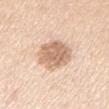This lesion was catalogued during total-body skin photography and was not selected for biopsy.
Cropped from a whole-body photographic skin survey; the tile spans about 15 mm.
Imaged with white-light lighting.
The lesion's longest dimension is about 4.5 mm.
A male subject, roughly 35 years of age.
The lesion is on the left upper arm.
Automated tile analysis of the lesion measured a lesion area of about 14 mm², an eccentricity of roughly 0.65, and a symmetry-axis asymmetry near 0.15. The software also gave a mean CIELAB color near L≈68 a*≈19 b*≈32, about 14 CIELAB-L* units darker than the surrounding skin, and a normalized border contrast of about 8. The software also gave a within-lesion color-variation index near 3.5/10 and radial color variation of about 1.5. And it measured a classifier nevus-likeness of about 20/100 and lesion-presence confidence of about 100/100.